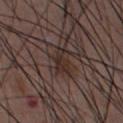{
  "patient": {
    "sex": "male",
    "age_approx": 50
  },
  "lesion_size": {
    "long_diameter_mm_approx": 3.5
  },
  "lighting": "white-light",
  "image": {
    "source": "total-body photography crop",
    "field_of_view_mm": 15
  },
  "site": "abdomen"
}